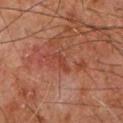{"biopsy_status": "not biopsied; imaged during a skin examination", "image": {"source": "total-body photography crop", "field_of_view_mm": 15}, "automated_metrics": {"color_variation_0_10": 0.0, "peripheral_color_asymmetry": 0.0, "nevus_likeness_0_100": 0}, "site": "upper back", "patient": {"sex": "male", "age_approx": 60}, "lighting": "cross-polarized"}